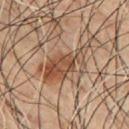{"biopsy_status": "not biopsied; imaged during a skin examination", "lesion_size": {"long_diameter_mm_approx": 7.0}, "lighting": "cross-polarized", "automated_metrics": {"color_variation_0_10": 5.5, "nevus_likeness_0_100": 90}, "image": {"source": "total-body photography crop", "field_of_view_mm": 15}, "patient": {"sex": "male", "age_approx": 50}, "site": "chest"}